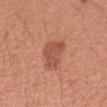* notes: catalogued during a skin exam; not biopsied